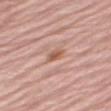biopsy status = total-body-photography surveillance lesion; no biopsy
patient = female, about 70 years old
location = the right thigh
TBP lesion metrics = an area of roughly 2.5 mm², an eccentricity of roughly 0.9, and a symmetry-axis asymmetry near 0.3; a classifier nevus-likeness of about 0/100 and a detector confidence of about 100 out of 100 that the crop contains a lesion
illumination = white-light illumination
size = ~2.5 mm (longest diameter)
imaging modality = ~15 mm tile from a whole-body skin photo